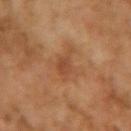Imaged during a routine full-body skin examination; the lesion was not biopsied and no histopathology is available.
Approximately 3.5 mm at its widest.
On the left upper arm.
A female patient aged 58–62.
This image is a 15 mm lesion crop taken from a total-body photograph.
Imaged with cross-polarized lighting.